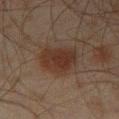Findings:
* notes · no biopsy performed (imaged during a skin exam)
* imaging modality · 15 mm crop, total-body photography
* site · the left thigh
* subject · male, approximately 45 years of age